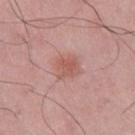<lesion>
<biopsy_status>not biopsied; imaged during a skin examination</biopsy_status>
<patient>
  <sex>male</sex>
  <age_approx>50</age_approx>
</patient>
<site>right upper arm</site>
<image>
  <source>total-body photography crop</source>
  <field_of_view_mm>15</field_of_view_mm>
</image>
</lesion>